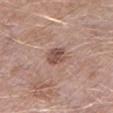• biopsy status · no biopsy performed (imaged during a skin exam)
• size · about 2.5 mm
• lighting · white-light
• imaging modality · 15 mm crop, total-body photography
• patient · male, aged 48 to 52
• location · the left lower leg